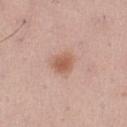  biopsy_status: not biopsied; imaged during a skin examination
  site: right thigh
  image:
    source: total-body photography crop
    field_of_view_mm: 15
  patient:
    sex: male
    age_approx: 40